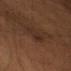notes: total-body-photography surveillance lesion; no biopsy
imaging modality: total-body-photography crop, ~15 mm field of view
body site: the left upper arm
subject: male, aged around 55
size: ~3 mm (longest diameter)
illumination: cross-polarized illumination
automated lesion analysis: a lesion color around L≈23 a*≈14 b*≈21 in CIELAB and a lesion-to-skin contrast of about 5 (normalized; higher = more distinct)